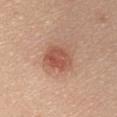| key | value |
|---|---|
| biopsy status | catalogued during a skin exam; not biopsied |
| diameter | ~4 mm (longest diameter) |
| image source | 15 mm crop, total-body photography |
| image-analysis metrics | a lesion color around L≈54 a*≈25 b*≈30 in CIELAB, a lesion–skin lightness drop of about 11, and a lesion-to-skin contrast of about 7.5 (normalized; higher = more distinct); a border-irregularity rating of about 2/10 and a within-lesion color-variation index near 5/10; a classifier nevus-likeness of about 100/100 and lesion-presence confidence of about 100/100 |
| location | the chest |
| subject | female, about 55 years old |
| illumination | white-light |